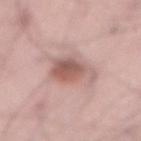Clinical impression:
No biopsy was performed on this lesion — it was imaged during a full skin examination and was not determined to be concerning.
Clinical summary:
Imaged with white-light lighting. From the abdomen. A male subject approximately 65 years of age. A 15 mm close-up extracted from a 3D total-body photography capture.